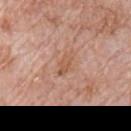Captured under white-light illumination. The recorded lesion diameter is about 2.5 mm. This image is a 15 mm lesion crop taken from a total-body photograph. Located on the chest. A male subject roughly 70 years of age.